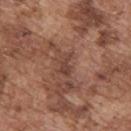Recorded during total-body skin imaging; not selected for excision or biopsy. Captured under white-light illumination. The lesion-visualizer software estimated a lesion–skin lightness drop of about 8 and a lesion-to-skin contrast of about 6 (normalized; higher = more distinct). And it measured border irregularity of about 5.5 on a 0–10 scale and radial color variation of about 0.5. It also reported a nevus-likeness score of about 0/100 and a detector confidence of about 85 out of 100 that the crop contains a lesion. A male subject, in their mid- to late 70s. From the upper back. Approximately 3 mm at its widest. A 15 mm close-up extracted from a 3D total-body photography capture.